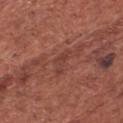{"biopsy_status": "not biopsied; imaged during a skin examination", "site": "head or neck", "patient": {"sex": "male", "age_approx": 65}, "lighting": "white-light", "automated_metrics": {"vs_skin_darker_L": 6.0, "vs_skin_contrast_norm": 5.0}, "image": {"source": "total-body photography crop", "field_of_view_mm": 15}}